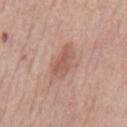Notes:
* location — the mid back
* patient — male, in their mid-70s
* lighting — white-light
* image — ~15 mm crop, total-body skin-cancer survey
* size — ≈4.5 mm